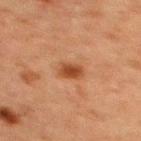biopsy status: total-body-photography surveillance lesion; no biopsy
body site: the upper back
subject: male, roughly 60 years of age
acquisition: 15 mm crop, total-body photography